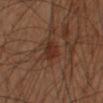The lesion is on the left forearm.
A 15 mm crop from a total-body photograph taken for skin-cancer surveillance.
Captured under cross-polarized illumination.
A male patient aged around 50.
Automated image analysis of the tile measured a nevus-likeness score of about 45/100 and a detector confidence of about 100 out of 100 that the crop contains a lesion.